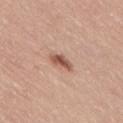follow-up: imaged on a skin check; not biopsied | tile lighting: white-light illumination | lesion size: ~3 mm (longest diameter) | subject: male, aged around 60 | acquisition: ~15 mm tile from a whole-body skin photo | location: the lower back.